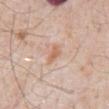workup — total-body-photography surveillance lesion; no biopsy | anatomic site — the abdomen | image — ~15 mm tile from a whole-body skin photo | diameter — about 2.5 mm | image-analysis metrics — a lesion area of about 3.5 mm² and an eccentricity of roughly 0.8 | subject — male, aged approximately 80 | illumination — white-light.